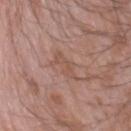Impression:
The lesion was photographed on a routine skin check and not biopsied; there is no pathology result.
Acquisition and patient details:
Cropped from a total-body skin-imaging series; the visible field is about 15 mm. On the right forearm. A male patient, roughly 60 years of age. Longest diameter approximately 3 mm. Captured under white-light illumination. Automated tile analysis of the lesion measured an average lesion color of about L≈52 a*≈20 b*≈27 (CIELAB) and a lesion–skin lightness drop of about 6.